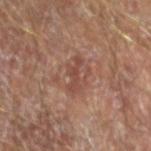Impression: Captured during whole-body skin photography for melanoma surveillance; the lesion was not biopsied. Image and clinical context: The total-body-photography lesion software estimated a lesion area of about 3.5 mm² and a symmetry-axis asymmetry near 0.4. The software also gave border irregularity of about 6 on a 0–10 scale, a color-variation rating of about 0/10, and a peripheral color-asymmetry measure near 0. The analysis additionally found a classifier nevus-likeness of about 0/100 and a lesion-detection confidence of about 100/100. A lesion tile, about 15 mm wide, cut from a 3D total-body photograph. The subject is a male aged around 65. Imaged with cross-polarized lighting. On the right forearm. The recorded lesion diameter is about 3.5 mm.